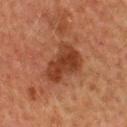TBP lesion metrics = an average lesion color of about L≈32 a*≈22 b*≈28 (CIELAB) and about 9 CIELAB-L* units darker than the surrounding skin; diameter = ≈5.5 mm; patient = female, aged 53–57; acquisition = ~15 mm tile from a whole-body skin photo; tile lighting = cross-polarized; body site = the back.